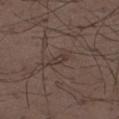Impression: This lesion was catalogued during total-body skin photography and was not selected for biopsy. Context: On the left thigh. The recorded lesion diameter is about 2.5 mm. The tile uses white-light illumination. Automated tile analysis of the lesion measured a footprint of about 2.5 mm², a shape eccentricity near 0.9, and a shape-asymmetry score of about 0.35 (0 = symmetric). And it measured an automated nevus-likeness rating near 0 out of 100 and lesion-presence confidence of about 70/100. A roughly 15 mm field-of-view crop from a total-body skin photograph. A male subject aged around 50.